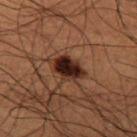No biopsy was performed on this lesion — it was imaged during a full skin examination and was not determined to be concerning.
Cropped from a whole-body photographic skin survey; the tile spans about 15 mm.
The patient is a male in their 50s.
Captured under cross-polarized illumination.
The lesion's longest dimension is about 3.5 mm.
The lesion is located on the left thigh.
Automated tile analysis of the lesion measured an eccentricity of roughly 0.7 and a symmetry-axis asymmetry near 0.2. It also reported a within-lesion color-variation index near 5/10 and a peripheral color-asymmetry measure near 1.5.